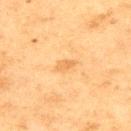Automated image analysis of the tile measured lesion-presence confidence of about 100/100.
Measured at roughly 2.5 mm in maximum diameter.
The patient is a male aged approximately 75.
Located on the upper back.
This is a cross-polarized tile.
A close-up tile cropped from a whole-body skin photograph, about 15 mm across.